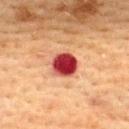Assessment:
Part of a total-body skin-imaging series; this lesion was reviewed on a skin check and was not flagged for biopsy.
Clinical summary:
Located on the upper back. The recorded lesion diameter is about 3.5 mm. The patient is a female in their mid- to late 50s. Captured under cross-polarized illumination. This image is a 15 mm lesion crop taken from a total-body photograph.